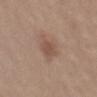Part of a total-body skin-imaging series; this lesion was reviewed on a skin check and was not flagged for biopsy. This image is a 15 mm lesion crop taken from a total-body photograph. A female patient, approximately 20 years of age. Captured under white-light illumination. Located on the lower back. The total-body-photography lesion software estimated an area of roughly 4.5 mm² and two-axis asymmetry of about 0.2. It also reported a within-lesion color-variation index near 1.5/10 and peripheral color asymmetry of about 0.5. And it measured a classifier nevus-likeness of about 20/100 and lesion-presence confidence of about 100/100.